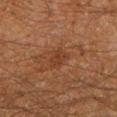notes: no biopsy performed (imaged during a skin exam)
TBP lesion metrics: a footprint of about 3 mm², an eccentricity of roughly 0.8, and two-axis asymmetry of about 0.25; a nevus-likeness score of about 0/100 and a detector confidence of about 100 out of 100 that the crop contains a lesion
site: the left lower leg
patient: male, about 60 years old
image: 15 mm crop, total-body photography
lighting: cross-polarized illumination